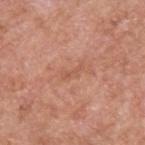Q: Was this lesion biopsied?
A: no biopsy performed (imaged during a skin exam)
Q: What is the anatomic site?
A: the upper back
Q: Patient demographics?
A: male, in their 60s
Q: Lesion size?
A: about 3 mm
Q: What is the imaging modality?
A: ~15 mm tile from a whole-body skin photo
Q: What did automated image analysis measure?
A: a lesion area of about 2.5 mm², a shape eccentricity near 0.95, and a symmetry-axis asymmetry near 0.55; about 6 CIELAB-L* units darker than the surrounding skin and a lesion-to-skin contrast of about 4.5 (normalized; higher = more distinct); a border-irregularity rating of about 6/10; a classifier nevus-likeness of about 0/100
Q: What lighting was used for the tile?
A: white-light illumination